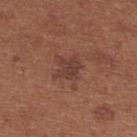Q: Is there a histopathology result?
A: imaged on a skin check; not biopsied
Q: Lesion location?
A: the back
Q: Who is the patient?
A: female, aged 63 to 67
Q: What lighting was used for the tile?
A: white-light illumination
Q: What kind of image is this?
A: ~15 mm tile from a whole-body skin photo
Q: Lesion size?
A: about 3 mm
Q: Automated lesion metrics?
A: a lesion area of about 6.5 mm², a shape eccentricity near 0.4, and two-axis asymmetry of about 0.3; an average lesion color of about L≈39 a*≈21 b*≈25 (CIELAB), a lesion–skin lightness drop of about 7, and a normalized border contrast of about 6.5; a border-irregularity rating of about 4.5/10, internal color variation of about 2 on a 0–10 scale, and radial color variation of about 1